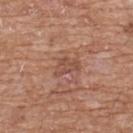Captured during whole-body skin photography for melanoma surveillance; the lesion was not biopsied.
This is a white-light tile.
Automated tile analysis of the lesion measured an outline eccentricity of about 0.8 (0 = round, 1 = elongated). It also reported a lesion color around L≈49 a*≈22 b*≈28 in CIELAB, roughly 8 lightness units darker than nearby skin, and a normalized border contrast of about 5.5. And it measured an automated nevus-likeness rating near 0 out of 100 and lesion-presence confidence of about 95/100.
A male patient, approximately 60 years of age.
Located on the front of the torso.
A 15 mm crop from a total-body photograph taken for skin-cancer surveillance.
The recorded lesion diameter is about 3 mm.